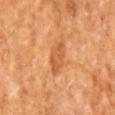This lesion was catalogued during total-body skin photography and was not selected for biopsy. A male patient aged approximately 65. Longest diameter approximately 5 mm. An algorithmic analysis of the crop reported a lesion color around L≈55 a*≈26 b*≈41 in CIELAB, about 9 CIELAB-L* units darker than the surrounding skin, and a normalized border contrast of about 6. The analysis additionally found border irregularity of about 3 on a 0–10 scale, a within-lesion color-variation index near 2.5/10, and peripheral color asymmetry of about 1. The analysis additionally found an automated nevus-likeness rating near 0 out of 100. From the mid back. This is a cross-polarized tile. A 15 mm close-up extracted from a 3D total-body photography capture.